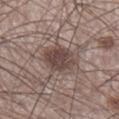Impression:
Imaged during a routine full-body skin examination; the lesion was not biopsied and no histopathology is available.
Clinical summary:
From the right lower leg. Cropped from a total-body skin-imaging series; the visible field is about 15 mm. The lesion's longest dimension is about 4.5 mm. Automated image analysis of the tile measured a mean CIELAB color near L≈44 a*≈15 b*≈20 and roughly 11 lightness units darker than nearby skin. The patient is a male aged approximately 60.